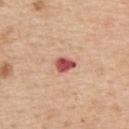biopsy status — catalogued during a skin exam; not biopsied | tile lighting — white-light illumination | body site — the upper back | patient — male, in their 60s | imaging modality — 15 mm crop, total-body photography.